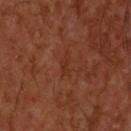The lesion was tiled from a total-body skin photograph and was not biopsied. The lesion is on the upper back. The tile uses cross-polarized illumination. An algorithmic analysis of the crop reported a lesion color around L≈31 a*≈24 b*≈30 in CIELAB, roughly 4 lightness units darker than nearby skin, and a lesion-to-skin contrast of about 5 (normalized; higher = more distinct). And it measured border irregularity of about 3.5 on a 0–10 scale, a color-variation rating of about 0/10, and radial color variation of about 0. It also reported lesion-presence confidence of about 100/100. The lesion's longest dimension is about 2.5 mm. A 15 mm close-up tile from a total-body photography series done for melanoma screening. A male patient about 55 years old.